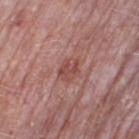  biopsy_status: not biopsied; imaged during a skin examination